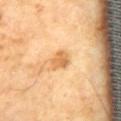  site: back
  image:
    source: total-body photography crop
    field_of_view_mm: 15
  patient:
    sex: male
    age_approx: 70